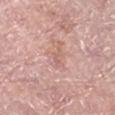Automated image analysis of the tile measured a lesion color around L≈61 a*≈22 b*≈26 in CIELAB, a lesion–skin lightness drop of about 7, and a normalized lesion–skin contrast near 4.5. The software also gave border irregularity of about 6 on a 0–10 scale and a color-variation rating of about 0/10. On the right lower leg. Approximately 2.5 mm at its widest. Captured under white-light illumination. A female patient, aged 58 to 62. A 15 mm crop from a total-body photograph taken for skin-cancer surveillance.